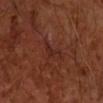Q: Is there a histopathology result?
A: catalogued during a skin exam; not biopsied
Q: Where on the body is the lesion?
A: the right forearm
Q: Automated lesion metrics?
A: an area of roughly 3 mm², an eccentricity of roughly 0.8, and a symmetry-axis asymmetry near 0.5; a mean CIELAB color near L≈24 a*≈21 b*≈23 and a normalized border contrast of about 5.5; border irregularity of about 5.5 on a 0–10 scale, internal color variation of about 1 on a 0–10 scale, and peripheral color asymmetry of about 0.5; lesion-presence confidence of about 95/100
Q: What is the imaging modality?
A: 15 mm crop, total-body photography
Q: Patient demographics?
A: male, aged around 60